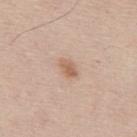Clinical impression: Captured during whole-body skin photography for melanoma surveillance; the lesion was not biopsied. Background: A 15 mm close-up extracted from a 3D total-body photography capture. This is a white-light tile. Approximately 2.5 mm at its widest. The lesion is on the mid back. A male patient approximately 45 years of age.